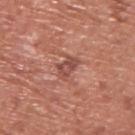No biopsy was performed on this lesion — it was imaged during a full skin examination and was not determined to be concerning. A male patient, in their mid- to late 70s. From the upper back. A region of skin cropped from a whole-body photographic capture, roughly 15 mm wide. Captured under white-light illumination.